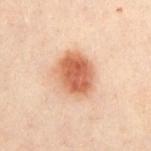Captured during whole-body skin photography for melanoma surveillance; the lesion was not biopsied. From the chest. This is a cross-polarized tile. Cropped from a whole-body photographic skin survey; the tile spans about 15 mm. An algorithmic analysis of the crop reported an average lesion color of about L≈51 a*≈22 b*≈30 (CIELAB) and a normalized lesion–skin contrast near 9.5. And it measured border irregularity of about 1.5 on a 0–10 scale, a color-variation rating of about 4.5/10, and a peripheral color-asymmetry measure near 1.5. A male patient, approximately 50 years of age.